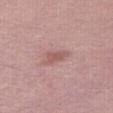No biopsy was performed on this lesion — it was imaged during a full skin examination and was not determined to be concerning.
About 3 mm across.
The subject is a female roughly 60 years of age.
Captured under white-light illumination.
A region of skin cropped from a whole-body photographic capture, roughly 15 mm wide.
Located on the right thigh.